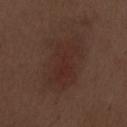workup = imaged on a skin check; not biopsied
illumination = white-light illumination
subject = male, roughly 70 years of age
site = the abdomen
size = ~7.5 mm (longest diameter)
image = 15 mm crop, total-body photography
automated metrics = an automated nevus-likeness rating near 70 out of 100